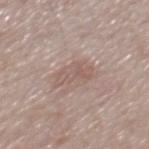The subject is a male aged around 75.
Cropped from a total-body skin-imaging series; the visible field is about 15 mm.
The lesion is on the mid back.
The tile uses white-light illumination.
Automated image analysis of the tile measured a mean CIELAB color near L≈56 a*≈17 b*≈22, about 7 CIELAB-L* units darker than the surrounding skin, and a lesion-to-skin contrast of about 5 (normalized; higher = more distinct). The software also gave a border-irregularity rating of about 5/10 and a within-lesion color-variation index near 3/10. The analysis additionally found a nevus-likeness score of about 0/100 and a detector confidence of about 100 out of 100 that the crop contains a lesion.
About 3.5 mm across.